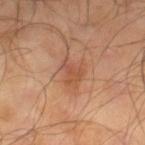Recorded during total-body skin imaging; not selected for excision or biopsy.
A male subject, in their mid-60s.
Automated image analysis of the tile measured a lesion area of about 3.5 mm² and an outline eccentricity of about 0.7 (0 = round, 1 = elongated). The software also gave an automated nevus-likeness rating near 10 out of 100.
A close-up tile cropped from a whole-body skin photograph, about 15 mm across.
Approximately 2.5 mm at its widest.
The lesion is located on the right lower leg.
The tile uses cross-polarized illumination.